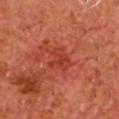Captured during whole-body skin photography for melanoma surveillance; the lesion was not biopsied.
A 15 mm crop from a total-body photograph taken for skin-cancer surveillance.
The subject is a male in their mid- to late 60s.
The tile uses cross-polarized illumination.
About 3 mm across.